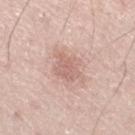Clinical impression:
Part of a total-body skin-imaging series; this lesion was reviewed on a skin check and was not flagged for biopsy.
Context:
A male patient, approximately 75 years of age. About 3.5 mm across. The lesion is located on the right thigh. The lesion-visualizer software estimated a lesion area of about 7 mm², an eccentricity of roughly 0.7, and a shape-asymmetry score of about 0.3 (0 = symmetric). It also reported a border-irregularity rating of about 3.5/10 and a peripheral color-asymmetry measure near 0.5. Cropped from a total-body skin-imaging series; the visible field is about 15 mm.